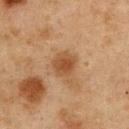follow-up: imaged on a skin check; not biopsied | subject: male, approximately 75 years of age | imaging modality: ~15 mm crop, total-body skin-cancer survey | size: about 3 mm | site: the chest.